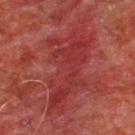workup=total-body-photography surveillance lesion; no biopsy
lighting=cross-polarized illumination
acquisition=~15 mm tile from a whole-body skin photo
patient=male, approximately 60 years of age
body site=the upper back
lesion diameter=about 10 mm
automated lesion analysis=an area of roughly 31 mm² and an eccentricity of roughly 0.9; a nevus-likeness score of about 0/100 and a lesion-detection confidence of about 70/100